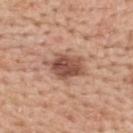<lesion>
  <lighting>white-light</lighting>
  <lesion_size>
    <long_diameter_mm_approx>5.0</long_diameter_mm_approx>
  </lesion_size>
  <site>upper back</site>
  <patient>
    <sex>female</sex>
    <age_approx>50</age_approx>
  </patient>
  <image>
    <source>total-body photography crop</source>
    <field_of_view_mm>15</field_of_view_mm>
  </image>
</lesion>